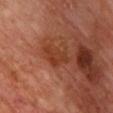Impression: Captured during whole-body skin photography for melanoma surveillance; the lesion was not biopsied. Image and clinical context: A male patient roughly 70 years of age. A lesion tile, about 15 mm wide, cut from a 3D total-body photograph. From the chest.